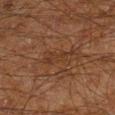| field | value |
|---|---|
| notes | catalogued during a skin exam; not biopsied |
| subject | male, about 60 years old |
| body site | the right lower leg |
| image-analysis metrics | a lesion color around L≈26 a*≈16 b*≈24 in CIELAB, a lesion–skin lightness drop of about 4, and a normalized border contrast of about 4.5 |
| tile lighting | cross-polarized |
| image source | total-body-photography crop, ~15 mm field of view |